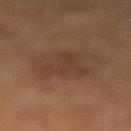Case summary:
• biopsy status — catalogued during a skin exam; not biopsied
• subject — male, about 45 years old
• TBP lesion metrics — a footprint of about 12 mm², an outline eccentricity of about 0.65 (0 = round, 1 = elongated), and a symmetry-axis asymmetry near 0.45; a mean CIELAB color near L≈37 a*≈17 b*≈28, a lesion–skin lightness drop of about 6, and a lesion-to-skin contrast of about 5 (normalized; higher = more distinct); a border-irregularity index near 5/10, a within-lesion color-variation index near 2.5/10, and radial color variation of about 1
• tile lighting — cross-polarized illumination
• lesion size — ~5 mm (longest diameter)
• acquisition — ~15 mm tile from a whole-body skin photo
• site — the left lower leg